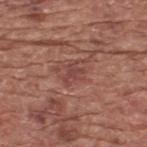The lesion was photographed on a routine skin check and not biopsied; there is no pathology result. The patient is a male about 75 years old. This is a white-light tile. On the upper back. An algorithmic analysis of the crop reported a shape eccentricity near 0.6 and two-axis asymmetry of about 0.4. It also reported a border-irregularity index near 5.5/10, internal color variation of about 2 on a 0–10 scale, and a peripheral color-asymmetry measure near 1. A lesion tile, about 15 mm wide, cut from a 3D total-body photograph. Measured at roughly 3 mm in maximum diameter.